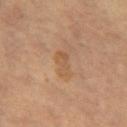Imaged during a routine full-body skin examination; the lesion was not biopsied and no histopathology is available.